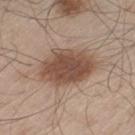Clinical impression:
No biopsy was performed on this lesion — it was imaged during a full skin examination and was not determined to be concerning.
Clinical summary:
On the left thigh. A male patient, approximately 60 years of age. A lesion tile, about 15 mm wide, cut from a 3D total-body photograph.